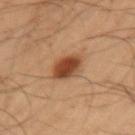Assessment: The lesion was tiled from a total-body skin photograph and was not biopsied. Context: Imaged with cross-polarized lighting. The subject is a male in their mid- to late 50s. Located on the left upper arm. An algorithmic analysis of the crop reported an area of roughly 8 mm² and two-axis asymmetry of about 0.15. The software also gave a border-irregularity index near 1/10, a color-variation rating of about 4.5/10, and radial color variation of about 1.5. A lesion tile, about 15 mm wide, cut from a 3D total-body photograph.